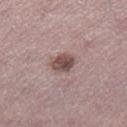{
  "biopsy_status": "not biopsied; imaged during a skin examination",
  "patient": {
    "sex": "female",
    "age_approx": 50
  },
  "automated_metrics": {
    "area_mm2_approx": 5.5,
    "eccentricity": 0.6,
    "shape_asymmetry": 0.2,
    "vs_skin_darker_L": 13.0,
    "vs_skin_contrast_norm": 9.5,
    "border_irregularity_0_10": 1.5,
    "color_variation_0_10": 4.0,
    "peripheral_color_asymmetry": 1.5
  },
  "lesion_size": {
    "long_diameter_mm_approx": 3.0
  },
  "lighting": "white-light",
  "site": "leg",
  "image": {
    "source": "total-body photography crop",
    "field_of_view_mm": 15
  }
}